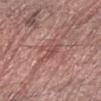Recorded during total-body skin imaging; not selected for excision or biopsy. Cropped from a whole-body photographic skin survey; the tile spans about 15 mm. On the arm. An algorithmic analysis of the crop reported an area of roughly 4.5 mm², a shape eccentricity near 0.65, and a shape-asymmetry score of about 0.5 (0 = symmetric). The analysis additionally found border irregularity of about 5 on a 0–10 scale, internal color variation of about 2.5 on a 0–10 scale, and peripheral color asymmetry of about 1. The patient is a male aged around 70. Imaged with white-light lighting.